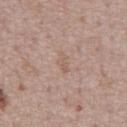This lesion was catalogued during total-body skin photography and was not selected for biopsy.
Imaged with white-light lighting.
Measured at roughly 2.5 mm in maximum diameter.
The lesion is located on the chest.
Automated tile analysis of the lesion measured internal color variation of about 0.5 on a 0–10 scale and radial color variation of about 0. It also reported lesion-presence confidence of about 100/100.
This image is a 15 mm lesion crop taken from a total-body photograph.
The subject is a male approximately 75 years of age.